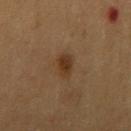<lesion>
<biopsy_status>not biopsied; imaged during a skin examination</biopsy_status>
<lesion_size>
  <long_diameter_mm_approx>2.5</long_diameter_mm_approx>
</lesion_size>
<image>
  <source>total-body photography crop</source>
  <field_of_view_mm>15</field_of_view_mm>
</image>
<lighting>cross-polarized</lighting>
<site>right upper arm</site>
<patient>
  <sex>male</sex>
  <age_approx>60</age_approx>
</patient>
</lesion>